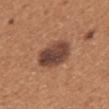| field | value |
|---|---|
| workup | total-body-photography surveillance lesion; no biopsy |
| lesion size | ~4.5 mm (longest diameter) |
| subject | female, aged around 35 |
| tile lighting | white-light |
| acquisition | total-body-photography crop, ~15 mm field of view |
| body site | the mid back |